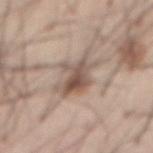<record>
  <biopsy_status>not biopsied; imaged during a skin examination</biopsy_status>
  <image>
    <source>total-body photography crop</source>
    <field_of_view_mm>15</field_of_view_mm>
  </image>
  <patient>
    <sex>male</sex>
    <age_approx>55</age_approx>
  </patient>
  <site>abdomen</site>
</record>